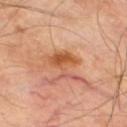  biopsy_status: not biopsied; imaged during a skin examination
  patient:
    sex: male
    age_approx: 70
  lighting: cross-polarized
  site: right thigh
  image:
    source: total-body photography crop
    field_of_view_mm: 15
  lesion_size:
    long_diameter_mm_approx: 4.0